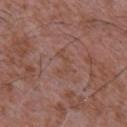Findings:
- diameter — ~3.5 mm (longest diameter)
- image source — ~15 mm crop, total-body skin-cancer survey
- patient — male, approximately 45 years of age
- site — the chest